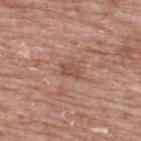<case>
<biopsy_status>not biopsied; imaged during a skin examination</biopsy_status>
<image>
  <source>total-body photography crop</source>
  <field_of_view_mm>15</field_of_view_mm>
</image>
<patient>
  <sex>male</sex>
  <age_approx>65</age_approx>
</patient>
<lighting>white-light</lighting>
<lesion_size>
  <long_diameter_mm_approx>2.5</long_diameter_mm_approx>
</lesion_size>
<site>upper back</site>
</case>